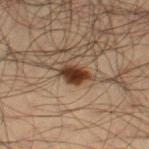No biopsy was performed on this lesion — it was imaged during a full skin examination and was not determined to be concerning. The patient is a male in their mid-30s. A close-up tile cropped from a whole-body skin photograph, about 15 mm across. On the right thigh. Captured under cross-polarized illumination. About 3.5 mm across.